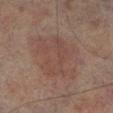Clinical impression:
The lesion was photographed on a routine skin check and not biopsied; there is no pathology result.